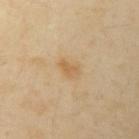Impression:
The lesion was photographed on a routine skin check and not biopsied; there is no pathology result.
Context:
A male patient aged 48–52. Imaged with cross-polarized lighting. A 15 mm close-up extracted from a 3D total-body photography capture. About 2.5 mm across. From the left upper arm.